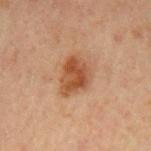The lesion was photographed on a routine skin check and not biopsied; there is no pathology result.
Automated image analysis of the tile measured a border-irregularity rating of about 2.5/10 and radial color variation of about 1.5. And it measured an automated nevus-likeness rating near 90 out of 100 and lesion-presence confidence of about 100/100.
A male patient, aged 43 to 47.
Approximately 4.5 mm at its widest.
A 15 mm close-up tile from a total-body photography series done for melanoma screening.
The lesion is located on the left upper arm.
The tile uses cross-polarized illumination.